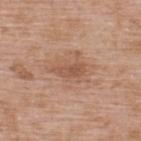Assessment:
Captured during whole-body skin photography for melanoma surveillance; the lesion was not biopsied.
Image and clinical context:
A close-up tile cropped from a whole-body skin photograph, about 15 mm across. The patient is a male approximately 50 years of age. From the upper back. This is a white-light tile. Measured at roughly 3.5 mm in maximum diameter.